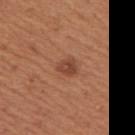Assessment: The lesion was photographed on a routine skin check and not biopsied; there is no pathology result. Clinical summary: Approximately 3.5 mm at its widest. On the arm. Captured under white-light illumination. The subject is a female approximately 65 years of age. The lesion-visualizer software estimated an area of roughly 7.5 mm², a shape eccentricity near 0.35, and two-axis asymmetry of about 0.2. A roughly 15 mm field-of-view crop from a total-body skin photograph.